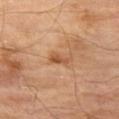Findings:
- patient — male, approximately 70 years of age
- imaging modality — ~15 mm tile from a whole-body skin photo
- illumination — cross-polarized
- anatomic site — the left thigh
- diameter — ~3 mm (longest diameter)